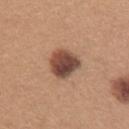The lesion was tiled from a total-body skin photograph and was not biopsied.
A 15 mm crop from a total-body photograph taken for skin-cancer surveillance.
The subject is a female aged approximately 30.
Located on the upper back.